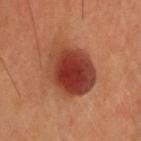A 15 mm close-up extracted from a 3D total-body photography capture.
A male subject, roughly 40 years of age.
Located on the head or neck.